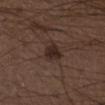Q: What are the patient's age and sex?
A: male, aged approximately 50
Q: Illumination type?
A: white-light
Q: How was this image acquired?
A: ~15 mm tile from a whole-body skin photo
Q: Where on the body is the lesion?
A: the right lower leg